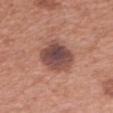Captured during whole-body skin photography for melanoma surveillance; the lesion was not biopsied.
The lesion is located on the left forearm.
The subject is a female aged around 40.
A 15 mm close-up extracted from a 3D total-body photography capture.
Approximately 4 mm at its widest.
An algorithmic analysis of the crop reported a mean CIELAB color near L≈46 a*≈22 b*≈22 and about 14 CIELAB-L* units darker than the surrounding skin.